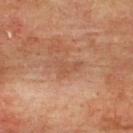Notes:
• biopsy status — catalogued during a skin exam; not biopsied
• anatomic site — the upper back
• illumination — cross-polarized
• patient — male, about 75 years old
• lesion diameter — ~3 mm (longest diameter)
• image — ~15 mm tile from a whole-body skin photo